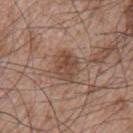Impression: Captured during whole-body skin photography for melanoma surveillance; the lesion was not biopsied. Image and clinical context: Imaged with white-light lighting. The lesion is on the left upper arm. A roughly 15 mm field-of-view crop from a total-body skin photograph. A male patient aged around 65. The lesion-visualizer software estimated a lesion color around L≈47 a*≈19 b*≈27 in CIELAB, about 9 CIELAB-L* units darker than the surrounding skin, and a normalized border contrast of about 7. It also reported a nevus-likeness score of about 5/100 and lesion-presence confidence of about 100/100. Longest diameter approximately 3.5 mm.